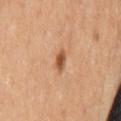Case summary:
* acquisition — 15 mm crop, total-body photography
* patient — female, in their 60s
* site — the lower back
* diameter — about 2.5 mm
* automated lesion analysis — an outline eccentricity of about 0.75 (0 = round, 1 = elongated) and two-axis asymmetry of about 0.2; an average lesion color of about L≈55 a*≈25 b*≈37 (CIELAB), about 13 CIELAB-L* units darker than the surrounding skin, and a normalized lesion–skin contrast near 8.5; border irregularity of about 1.5 on a 0–10 scale and radial color variation of about 0.5